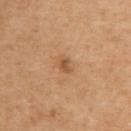Recorded during total-body skin imaging; not selected for excision or biopsy. From the back. A close-up tile cropped from a whole-body skin photograph, about 15 mm across. The tile uses cross-polarized illumination. Longest diameter approximately 2 mm. A female subject, in their 50s.